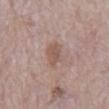biopsy status = catalogued during a skin exam; not biopsied | patient = male, in their mid-60s | body site = the mid back | acquisition = ~15 mm crop, total-body skin-cancer survey.